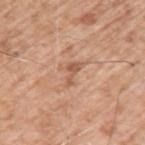Case summary:
- workup: total-body-photography surveillance lesion; no biopsy
- size: about 3 mm
- image: total-body-photography crop, ~15 mm field of view
- illumination: white-light
- location: the left upper arm
- patient: male, aged around 60